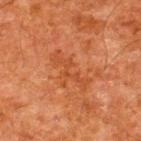anatomic site: the upper back; size: ≈5.5 mm; image: ~15 mm crop, total-body skin-cancer survey; patient: male, approximately 60 years of age; tile lighting: cross-polarized.